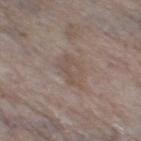This is a white-light tile. Cropped from a total-body skin-imaging series; the visible field is about 15 mm. The lesion's longest dimension is about 4.5 mm. A female patient roughly 85 years of age or older. On the right thigh.